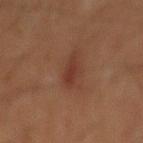Q: Was a biopsy performed?
A: no biopsy performed (imaged during a skin exam)
Q: What is the imaging modality?
A: 15 mm crop, total-body photography
Q: How was the tile lit?
A: cross-polarized illumination
Q: What is the anatomic site?
A: the abdomen
Q: What are the patient's age and sex?
A: male, approximately 60 years of age
Q: Lesion size?
A: ≈4 mm